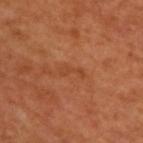Impression: No biopsy was performed on this lesion — it was imaged during a full skin examination and was not determined to be concerning. Image and clinical context: Longest diameter approximately 3 mm. The patient is a male aged 48–52. A roughly 15 mm field-of-view crop from a total-body skin photograph. The tile uses cross-polarized illumination. The lesion is located on the upper back.